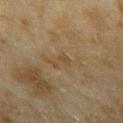Findings:
– follow-up · catalogued during a skin exam; not biopsied
– subject · female, in their 60s
– image · ~15 mm tile from a whole-body skin photo
– lighting · cross-polarized
– lesion diameter · ~3 mm (longest diameter)
– anatomic site · the right forearm
– TBP lesion metrics · an area of roughly 2.5 mm², an outline eccentricity of about 0.9 (0 = round, 1 = elongated), and a shape-asymmetry score of about 0.4 (0 = symmetric); border irregularity of about 5 on a 0–10 scale and radial color variation of about 0; an automated nevus-likeness rating near 0 out of 100